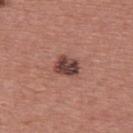{"biopsy_status": "not biopsied; imaged during a skin examination", "site": "upper back", "patient": {"sex": "male", "age_approx": 40}, "image": {"source": "total-body photography crop", "field_of_view_mm": 15}}